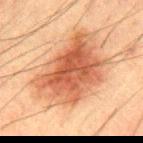| feature | finding |
|---|---|
| follow-up | catalogued during a skin exam; not biopsied |
| lesion diameter | ~9.5 mm (longest diameter) |
| tile lighting | cross-polarized |
| imaging modality | total-body-photography crop, ~15 mm field of view |
| body site | the mid back |
| patient | male, in their 50s |